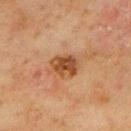Q: Was this lesion biopsied?
A: total-body-photography surveillance lesion; no biopsy
Q: What kind of image is this?
A: total-body-photography crop, ~15 mm field of view
Q: What is the anatomic site?
A: the back
Q: What is the lesion's diameter?
A: ~3.5 mm (longest diameter)
Q: How was the tile lit?
A: cross-polarized illumination
Q: Patient demographics?
A: male, aged 68 to 72
Q: What did automated image analysis measure?
A: a footprint of about 7.5 mm² and a shape eccentricity near 0.6; a lesion color around L≈40 a*≈21 b*≈32 in CIELAB, roughly 11 lightness units darker than nearby skin, and a normalized lesion–skin contrast near 9; a border-irregularity index near 2/10 and a color-variation rating of about 4/10; lesion-presence confidence of about 100/100